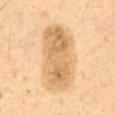* lighting: cross-polarized
* acquisition: total-body-photography crop, ~15 mm field of view
* patient: male, approximately 60 years of age
* automated lesion analysis: an area of roughly 28 mm², an outline eccentricity of about 0.9 (0 = round, 1 = elongated), and a shape-asymmetry score of about 0.1 (0 = symmetric); border irregularity of about 2 on a 0–10 scale, a color-variation rating of about 4.5/10, and peripheral color asymmetry of about 1.5
* site: the abdomen